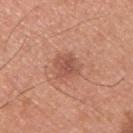Case summary:
- notes · total-body-photography surveillance lesion; no biopsy
- lesion diameter · ≈3 mm
- patient · male, roughly 30 years of age
- location · the upper back
- automated metrics · an average lesion color of about L≈53 a*≈25 b*≈30 (CIELAB) and a normalized border contrast of about 6.5
- illumination · white-light
- acquisition · total-body-photography crop, ~15 mm field of view